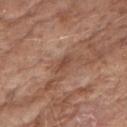follow-up = total-body-photography surveillance lesion; no biopsy.